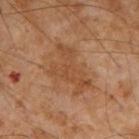<lesion>
<biopsy_status>not biopsied; imaged during a skin examination</biopsy_status>
<site>right upper arm</site>
<image>
  <source>total-body photography crop</source>
  <field_of_view_mm>15</field_of_view_mm>
</image>
<lesion_size>
  <long_diameter_mm_approx>6.0</long_diameter_mm_approx>
</lesion_size>
<lighting>cross-polarized</lighting>
<patient>
  <sex>male</sex>
  <age_approx>55</age_approx>
</patient>
<automated_metrics>
  <area_mm2_approx>15.0</area_mm2_approx>
  <eccentricity>0.75</eccentricity>
  <shape_asymmetry>0.5</shape_asymmetry>
  <border_irregularity_0_10>7.5</border_irregularity_0_10>
  <nevus_likeness_0_100>0</nevus_likeness_0_100>
  <lesion_detection_confidence_0_100>100</lesion_detection_confidence_0_100>
</automated_metrics>
</lesion>